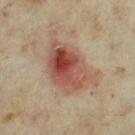Q: Was a biopsy performed?
A: no biopsy performed (imaged during a skin exam)